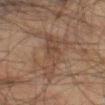A roughly 15 mm field-of-view crop from a total-body skin photograph. This is a cross-polarized tile. An algorithmic analysis of the crop reported a lesion–skin lightness drop of about 5 and a normalized border contrast of about 5. And it measured border irregularity of about 8 on a 0–10 scale and a within-lesion color-variation index near 3/10. A male patient aged approximately 65. The lesion is on the left thigh. The recorded lesion diameter is about 7.5 mm.